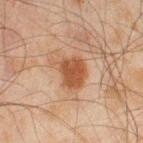Imaged during a routine full-body skin examination; the lesion was not biopsied and no histopathology is available.
About 4.5 mm across.
The patient is a male in their mid-40s.
A 15 mm close-up tile from a total-body photography series done for melanoma screening.
From the right thigh.